A female subject aged around 65 · a lesion tile, about 15 mm wide, cut from a 3D total-body photograph · located on the chest.
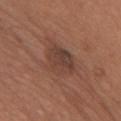The tile uses white-light illumination.
Automated tile analysis of the lesion measured a footprint of about 11 mm², an eccentricity of roughly 0.7, and a symmetry-axis asymmetry near 0.2. The analysis additionally found an average lesion color of about L≈41 a*≈19 b*≈25 (CIELAB), a lesion–skin lightness drop of about 8, and a normalized lesion–skin contrast near 7. It also reported a border-irregularity index near 2/10, a color-variation rating of about 4/10, and a peripheral color-asymmetry measure near 1.
About 4.5 mm across.
Biopsy histopathology demonstrated a seborrheic keratosis (benign).